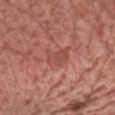notes = imaged on a skin check; not biopsied | automated lesion analysis = a lesion area of about 5 mm² and two-axis asymmetry of about 0.3; a lesion color around L≈49 a*≈28 b*≈28 in CIELAB, a lesion–skin lightness drop of about 7, and a normalized border contrast of about 5; a border-irregularity rating of about 4/10 and a peripheral color-asymmetry measure near 0.5; a nevus-likeness score of about 0/100 and a lesion-detection confidence of about 95/100 | tile lighting = white-light illumination | lesion diameter = ≈3 mm | location = the chest | subject = male, aged 73 to 77 | imaging modality = ~15 mm tile from a whole-body skin photo.